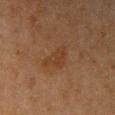Located on the arm.
The subject is a female roughly 60 years of age.
Captured under cross-polarized illumination.
About 4 mm across.
A 15 mm close-up extracted from a 3D total-body photography capture.